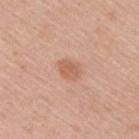Clinical impression: Captured during whole-body skin photography for melanoma surveillance; the lesion was not biopsied. Image and clinical context: The lesion is on the right upper arm. A male subject, aged 43–47. The total-body-photography lesion software estimated a lesion color around L≈60 a*≈24 b*≈32 in CIELAB and a lesion-to-skin contrast of about 6 (normalized; higher = more distinct). The analysis additionally found internal color variation of about 1.5 on a 0–10 scale. Cropped from a total-body skin-imaging series; the visible field is about 15 mm. The tile uses white-light illumination. Approximately 2.5 mm at its widest.